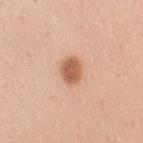Case summary:
* notes — catalogued during a skin exam; not biopsied
* patient — female, in their mid-30s
* image-analysis metrics — an eccentricity of roughly 0.65 and a symmetry-axis asymmetry near 0.2; a lesion color around L≈60 a*≈24 b*≈34 in CIELAB, roughly 14 lightness units darker than nearby skin, and a normalized lesion–skin contrast near 9; border irregularity of about 1.5 on a 0–10 scale, a color-variation rating of about 3/10, and a peripheral color-asymmetry measure near 1; lesion-presence confidence of about 100/100
* location — the right upper arm
* size — ~3 mm (longest diameter)
* acquisition — 15 mm crop, total-body photography